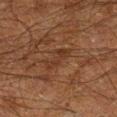Imaged during a routine full-body skin examination; the lesion was not biopsied and no histopathology is available. Captured under cross-polarized illumination. A male subject, aged around 60. A close-up tile cropped from a whole-body skin photograph, about 15 mm across. The lesion is on the leg. About 3.5 mm across.